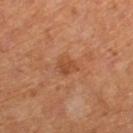Impression:
This lesion was catalogued during total-body skin photography and was not selected for biopsy.
Context:
This image is a 15 mm lesion crop taken from a total-body photograph. A female patient, roughly 65 years of age. From the leg.